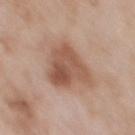- notes · imaged on a skin check; not biopsied
- site · the chest
- illumination · white-light illumination
- image · 15 mm crop, total-body photography
- patient · female, aged 58 to 62